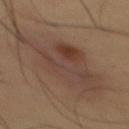Q: Is there a histopathology result?
A: catalogued during a skin exam; not biopsied
Q: Lesion size?
A: about 13 mm
Q: Illumination type?
A: cross-polarized
Q: What is the imaging modality?
A: total-body-photography crop, ~15 mm field of view
Q: Lesion location?
A: the chest
Q: Who is the patient?
A: male, approximately 55 years of age